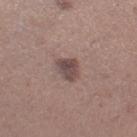{"biopsy_status": "not biopsied; imaged during a skin examination", "lesion_size": {"long_diameter_mm_approx": 3.0}, "patient": {"sex": "female", "age_approx": 30}, "image": {"source": "total-body photography crop", "field_of_view_mm": 15}, "automated_metrics": {"area_mm2_approx": 5.0, "eccentricity": 0.65, "shape_asymmetry": 0.35, "cielab_L": 46, "cielab_a": 17, "cielab_b": 18, "vs_skin_darker_L": 11.0, "vs_skin_contrast_norm": 8.5, "border_irregularity_0_10": 3.5, "color_variation_0_10": 3.5, "peripheral_color_asymmetry": 1.5}, "site": "leg"}